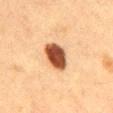<tbp_lesion>
  <biopsy_status>not biopsied; imaged during a skin examination</biopsy_status>
  <lighting>cross-polarized</lighting>
  <automated_metrics>
    <eccentricity>0.85</eccentricity>
    <shape_asymmetry>0.15</shape_asymmetry>
    <border_irregularity_0_10>2.0</border_irregularity_0_10>
    <color_variation_0_10>4.5</color_variation_0_10>
    <peripheral_color_asymmetry>1.5</peripheral_color_asymmetry>
  </automated_metrics>
  <patient>
    <sex>female</sex>
    <age_approx>40</age_approx>
  </patient>
  <lesion_size>
    <long_diameter_mm_approx>4.5</long_diameter_mm_approx>
  </lesion_size>
  <site>mid back</site>
  <image>
    <source>total-body photography crop</source>
    <field_of_view_mm>15</field_of_view_mm>
  </image>
</tbp_lesion>